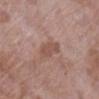This lesion was catalogued during total-body skin photography and was not selected for biopsy.
Cropped from a whole-body photographic skin survey; the tile spans about 15 mm.
From the left lower leg.
The tile uses white-light illumination.
A female subject, aged 68 to 72.
Longest diameter approximately 3.5 mm.